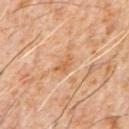{"automated_metrics": {"eccentricity": 0.8, "shape_asymmetry": 0.4}, "patient": {"sex": "male", "age_approx": 60}, "site": "chest", "lesion_size": {"long_diameter_mm_approx": 3.0}, "lighting": "cross-polarized", "image": {"source": "total-body photography crop", "field_of_view_mm": 15}}